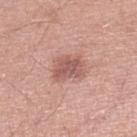biopsy status: imaged on a skin check; not biopsied | tile lighting: white-light | image source: 15 mm crop, total-body photography | anatomic site: the left lower leg | subject: male, aged approximately 45.